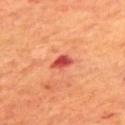notes: total-body-photography surveillance lesion; no biopsy
patient: male, about 70 years old
image: total-body-photography crop, ~15 mm field of view
lesion size: ~2.5 mm (longest diameter)
location: the upper back